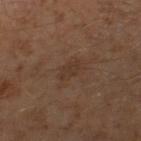<tbp_lesion>
<biopsy_status>not biopsied; imaged during a skin examination</biopsy_status>
<image>
  <source>total-body photography crop</source>
  <field_of_view_mm>15</field_of_view_mm>
</image>
<site>right thigh</site>
<patient>
  <sex>male</sex>
  <age_approx>60</age_approx>
</patient>
</tbp_lesion>